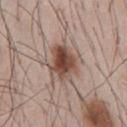Cropped from a whole-body photographic skin survey; the tile spans about 15 mm. A male patient, aged 53 to 57. The lesion-visualizer software estimated a lesion–skin lightness drop of about 14 and a normalized border contrast of about 9.5.